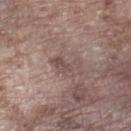biopsy status: imaged on a skin check; not biopsied
lesion diameter: ≈3.5 mm
patient: male, in their mid-70s
image source: 15 mm crop, total-body photography
body site: the left lower leg
TBP lesion metrics: an average lesion color of about L≈49 a*≈16 b*≈20 (CIELAB), roughly 8 lightness units darker than nearby skin, and a lesion-to-skin contrast of about 6 (normalized; higher = more distinct); a classifier nevus-likeness of about 0/100